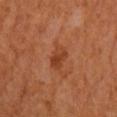Findings:
* follow-up — no biopsy performed (imaged during a skin exam)
* patient — female, about 65 years old
* acquisition — total-body-photography crop, ~15 mm field of view
* anatomic site — the right forearm